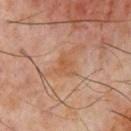Impression:
Imaged during a routine full-body skin examination; the lesion was not biopsied and no histopathology is available.
Image and clinical context:
Imaged with cross-polarized lighting. A male subject aged 58–62. Cropped from a total-body skin-imaging series; the visible field is about 15 mm. Automated tile analysis of the lesion measured an area of roughly 4 mm², an outline eccentricity of about 0.6 (0 = round, 1 = elongated), and a shape-asymmetry score of about 0.35 (0 = symmetric). It also reported a mean CIELAB color near L≈51 a*≈21 b*≈34 and a lesion-to-skin contrast of about 6 (normalized; higher = more distinct). The lesion is located on the chest.